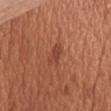Imaged with white-light lighting. The patient is a male aged approximately 65. The recorded lesion diameter is about 3 mm. A 15 mm close-up extracted from a 3D total-body photography capture. Located on the chest.